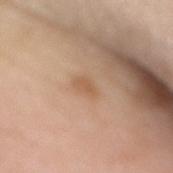Automated image analysis of the tile measured roughly 7 lightness units darker than nearby skin and a normalized lesion–skin contrast near 5. The analysis additionally found a border-irregularity rating of about 2/10, a color-variation rating of about 1/10, and peripheral color asymmetry of about 0.5.
The recorded lesion diameter is about 3 mm.
The lesion is located on the mid back.
A roughly 15 mm field-of-view crop from a total-body skin photograph.
This is a white-light tile.
A female patient, approximately 70 years of age.